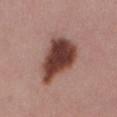workup = imaged on a skin check; not biopsied | anatomic site = the abdomen | acquisition = total-body-photography crop, ~15 mm field of view | subject = female, aged approximately 55 | image-analysis metrics = a lesion area of about 21 mm² and a shape eccentricity near 0.8; a border-irregularity index near 3/10, a within-lesion color-variation index near 6/10, and a peripheral color-asymmetry measure near 1.5; a detector confidence of about 100 out of 100 that the crop contains a lesion.